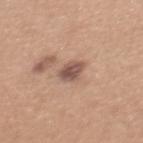Assessment: No biopsy was performed on this lesion — it was imaged during a full skin examination and was not determined to be concerning. Image and clinical context: From the back. Captured under white-light illumination. A female subject, aged 28–32. The lesion's longest dimension is about 2.5 mm. A lesion tile, about 15 mm wide, cut from a 3D total-body photograph.